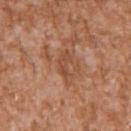biopsy_status: not biopsied; imaged during a skin examination
site: left upper arm
image:
  source: total-body photography crop
  field_of_view_mm: 15
lighting: white-light
patient:
  sex: male
  age_approx: 45